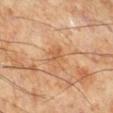follow-up=imaged on a skin check; not biopsied | subject=male, in their 70s | site=the right lower leg | lighting=cross-polarized | size=≈3 mm | image source=total-body-photography crop, ~15 mm field of view.